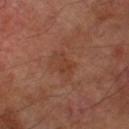Impression:
Part of a total-body skin-imaging series; this lesion was reviewed on a skin check and was not flagged for biopsy.
Background:
This is a cross-polarized tile. The lesion is on the leg. A male subject roughly 70 years of age. Longest diameter approximately 2.5 mm. Cropped from a whole-body photographic skin survey; the tile spans about 15 mm.